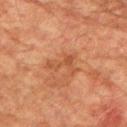Part of a total-body skin-imaging series; this lesion was reviewed on a skin check and was not flagged for biopsy.
A 15 mm crop from a total-body photograph taken for skin-cancer surveillance.
An algorithmic analysis of the crop reported a shape eccentricity near 0.85 and a symmetry-axis asymmetry near 0.7. It also reported a mean CIELAB color near L≈43 a*≈23 b*≈33, roughly 6 lightness units darker than nearby skin, and a normalized border contrast of about 5.5. It also reported a border-irregularity rating of about 9/10.
A male patient, in their mid-70s.
Located on the arm.